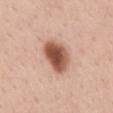Impression: Part of a total-body skin-imaging series; this lesion was reviewed on a skin check and was not flagged for biopsy. Image and clinical context: About 4.5 mm across. A 15 mm crop from a total-body photograph taken for skin-cancer surveillance. The patient is a female roughly 35 years of age. Located on the mid back. An algorithmic analysis of the crop reported a lesion area of about 11 mm² and an eccentricity of roughly 0.75. The analysis additionally found an automated nevus-likeness rating near 100 out of 100 and lesion-presence confidence of about 100/100. The tile uses white-light illumination.